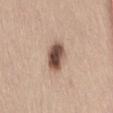Findings:
- biopsy status: imaged on a skin check; not biopsied
- tile lighting: white-light illumination
- body site: the lower back
- image: ~15 mm tile from a whole-body skin photo
- subject: female, aged 48–52
- lesion diameter: ≈3.5 mm